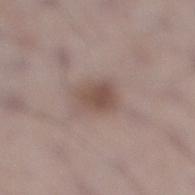Q: What kind of image is this?
A: 15 mm crop, total-body photography
Q: What is the anatomic site?
A: the leg
Q: Automated lesion metrics?
A: a lesion area of about 7.5 mm², an outline eccentricity of about 0.7 (0 = round, 1 = elongated), and two-axis asymmetry of about 0.2
Q: Who is the patient?
A: male, aged 68–72
Q: What lighting was used for the tile?
A: white-light
Q: How large is the lesion?
A: ~3.5 mm (longest diameter)